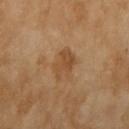<lesion>
<biopsy_status>not biopsied; imaged during a skin examination</biopsy_status>
<lesion_size>
  <long_diameter_mm_approx>4.0</long_diameter_mm_approx>
</lesion_size>
<image>
  <source>total-body photography crop</source>
  <field_of_view_mm>15</field_of_view_mm>
</image>
<site>arm</site>
<lighting>cross-polarized</lighting>
<patient>
  <sex>female</sex>
  <age_approx>70</age_approx>
</patient>
</lesion>